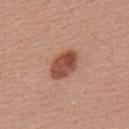notes — total-body-photography surveillance lesion; no biopsy
image — 15 mm crop, total-body photography
automated lesion analysis — a mean CIELAB color near L≈49 a*≈25 b*≈30, roughly 14 lightness units darker than nearby skin, and a normalized border contrast of about 9.5; a border-irregularity rating of about 1.5/10 and radial color variation of about 2; a nevus-likeness score of about 100/100 and lesion-presence confidence of about 100/100
site — the back
patient — male, approximately 55 years of age
illumination — white-light illumination